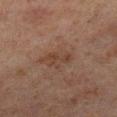Case summary:
- notes · imaged on a skin check; not biopsied
- patient · male, aged 68 to 72
- imaging modality · total-body-photography crop, ~15 mm field of view
- anatomic site · the left lower leg